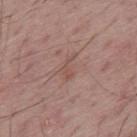tile lighting = white-light | lesion diameter = ~2.5 mm (longest diameter) | automated lesion analysis = a shape eccentricity near 0.85 and a symmetry-axis asymmetry near 0.55; a lesion color around L≈51 a*≈20 b*≈24 in CIELAB, about 6 CIELAB-L* units darker than the surrounding skin, and a normalized lesion–skin contrast near 5; a lesion-detection confidence of about 100/100 | location = the upper back | image source = ~15 mm tile from a whole-body skin photo | patient = male, aged 63–67.